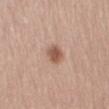This lesion was catalogued during total-body skin photography and was not selected for biopsy. Approximately 2.5 mm at its widest. A male subject approximately 65 years of age. Cropped from a total-body skin-imaging series; the visible field is about 15 mm. On the left thigh.